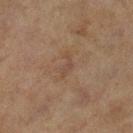The lesion was tiled from a total-body skin photograph and was not biopsied.